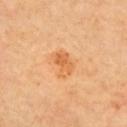No biopsy was performed on this lesion — it was imaged during a full skin examination and was not determined to be concerning. Imaged with cross-polarized lighting. From the right upper arm. The recorded lesion diameter is about 3.5 mm. A male subject, roughly 65 years of age. This image is a 15 mm lesion crop taken from a total-body photograph. Automated tile analysis of the lesion measured a lesion area of about 6 mm². And it measured an automated nevus-likeness rating near 50 out of 100 and lesion-presence confidence of about 100/100.